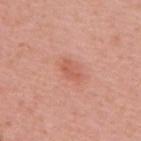Case summary:
- notes — total-body-photography surveillance lesion; no biopsy
- image source — 15 mm crop, total-body photography
- body site — the upper back
- size — ≈3 mm
- illumination — white-light illumination
- subject — male, aged around 60
- TBP lesion metrics — an area of roughly 3.5 mm², an eccentricity of roughly 0.85, and a symmetry-axis asymmetry near 0.35; a border-irregularity rating of about 3.5/10 and internal color variation of about 1.5 on a 0–10 scale